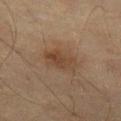{"biopsy_status": "not biopsied; imaged during a skin examination", "image": {"source": "total-body photography crop", "field_of_view_mm": 15}, "patient": {"sex": "female", "age_approx": 55}, "site": "leg"}